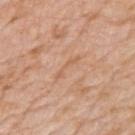The lesion was photographed on a routine skin check and not biopsied; there is no pathology result.